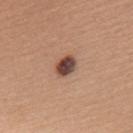| key | value |
|---|---|
| follow-up | imaged on a skin check; not biopsied |
| imaging modality | total-body-photography crop, ~15 mm field of view |
| lesion diameter | ≈3 mm |
| location | the back |
| patient | female, aged around 65 |
| lighting | white-light illumination |